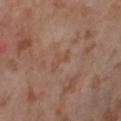The lesion was photographed on a routine skin check and not biopsied; there is no pathology result. Imaged with cross-polarized lighting. Longest diameter approximately 3 mm. The patient is a female aged 53 to 57. A close-up tile cropped from a whole-body skin photograph, about 15 mm across. On the left thigh.